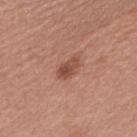Imaged during a routine full-body skin examination; the lesion was not biopsied and no histopathology is available. An algorithmic analysis of the crop reported a shape eccentricity near 0.8 and a symmetry-axis asymmetry near 0.2. The analysis additionally found a mean CIELAB color near L≈49 a*≈24 b*≈29, about 10 CIELAB-L* units darker than the surrounding skin, and a lesion-to-skin contrast of about 7.5 (normalized; higher = more distinct). The software also gave border irregularity of about 2.5 on a 0–10 scale, a within-lesion color-variation index near 4.5/10, and peripheral color asymmetry of about 2. The software also gave a nevus-likeness score of about 70/100 and a detector confidence of about 100 out of 100 that the crop contains a lesion. From the left upper arm. About 3 mm across. A close-up tile cropped from a whole-body skin photograph, about 15 mm across. A female patient, aged 38 to 42.